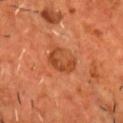Impression: Captured during whole-body skin photography for melanoma surveillance; the lesion was not biopsied. Acquisition and patient details: The lesion is on the chest. A 15 mm close-up extracted from a 3D total-body photography capture. The subject is a male about 55 years old.